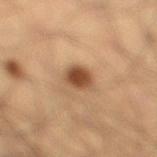The subject is a male in their mid- to late 30s. A 15 mm close-up extracted from a 3D total-body photography capture. Located on the leg.